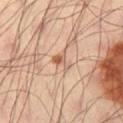Clinical impression: Captured during whole-body skin photography for melanoma surveillance; the lesion was not biopsied. Clinical summary: Located on the left thigh. A 15 mm crop from a total-body photograph taken for skin-cancer surveillance. A male subject, aged 38–42. Captured under cross-polarized illumination. An algorithmic analysis of the crop reported a shape eccentricity near 0.85. The analysis additionally found border irregularity of about 4 on a 0–10 scale and a within-lesion color-variation index near 3/10. And it measured a nevus-likeness score of about 25/100 and a lesion-detection confidence of about 100/100. The lesion's longest dimension is about 3 mm.